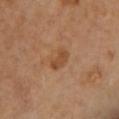Q: Was this lesion biopsied?
A: no biopsy performed (imaged during a skin exam)
Q: Automated lesion metrics?
A: a mean CIELAB color near L≈48 a*≈22 b*≈36, a lesion–skin lightness drop of about 8, and a normalized lesion–skin contrast near 7; a classifier nevus-likeness of about 0/100 and lesion-presence confidence of about 100/100
Q: How large is the lesion?
A: ≈3 mm
Q: Patient demographics?
A: female, aged approximately 55
Q: Lesion location?
A: the front of the torso
Q: How was this image acquired?
A: ~15 mm crop, total-body skin-cancer survey